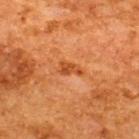– subject: male, aged approximately 65
– lesion size: ~2.5 mm (longest diameter)
– automated lesion analysis: a mean CIELAB color near L≈41 a*≈27 b*≈38 and roughly 9 lightness units darker than nearby skin
– tile lighting: cross-polarized
– site: the back
– image source: ~15 mm tile from a whole-body skin photo